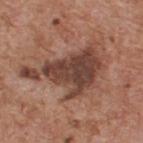• biopsy status — total-body-photography surveillance lesion; no biopsy
• acquisition — 15 mm crop, total-body photography
• TBP lesion metrics — a mean CIELAB color near L≈43 a*≈20 b*≈25 and roughly 13 lightness units darker than nearby skin; internal color variation of about 4 on a 0–10 scale and a peripheral color-asymmetry measure near 1; an automated nevus-likeness rating near 25 out of 100
• location — the upper back
• patient — male, aged 58–62